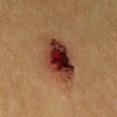This lesion was catalogued during total-body skin photography and was not selected for biopsy.
Measured at roughly 6.5 mm in maximum diameter.
On the abdomen.
An algorithmic analysis of the crop reported an average lesion color of about L≈30 a*≈20 b*≈25 (CIELAB), about 14 CIELAB-L* units darker than the surrounding skin, and a normalized lesion–skin contrast near 13.5. It also reported an automated nevus-likeness rating near 15 out of 100 and a detector confidence of about 100 out of 100 that the crop contains a lesion.
A 15 mm close-up extracted from a 3D total-body photography capture.
A female subject, in their mid-50s.